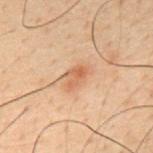biopsy_status: not biopsied; imaged during a skin examination
lighting: cross-polarized
site: mid back
automated_metrics:
  area_mm2_approx: 4.5
  eccentricity: 0.75
  shape_asymmetry: 0.3
  border_irregularity_0_10: 3.0
  color_variation_0_10: 3.5
  peripheral_color_asymmetry: 1.0
  nevus_likeness_0_100: 45
  lesion_detection_confidence_0_100: 100
image:
  source: total-body photography crop
  field_of_view_mm: 15
lesion_size:
  long_diameter_mm_approx: 3.0
patient:
  sex: male
  age_approx: 50